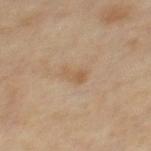{"site": "mid back", "image": {"source": "total-body photography crop", "field_of_view_mm": 15}, "automated_metrics": {"vs_skin_darker_L": 6.0, "vs_skin_contrast_norm": 5.0, "border_irregularity_0_10": 3.0, "color_variation_0_10": 2.5, "peripheral_color_asymmetry": 1.0, "nevus_likeness_0_100": 0, "lesion_detection_confidence_0_100": 100}, "lighting": "cross-polarized", "lesion_size": {"long_diameter_mm_approx": 3.0}, "patient": {"sex": "male", "age_approx": 55}}